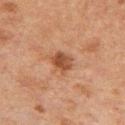Q: Was this lesion biopsied?
A: imaged on a skin check; not biopsied
Q: What is the anatomic site?
A: the arm
Q: What lighting was used for the tile?
A: cross-polarized illumination
Q: Patient demographics?
A: male, in their 50s
Q: What kind of image is this?
A: ~15 mm tile from a whole-body skin photo
Q: What is the lesion's diameter?
A: ≈3.5 mm